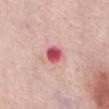* biopsy status: no biopsy performed (imaged during a skin exam)
* subject: female, in their mid-60s
* anatomic site: the front of the torso
* image source: 15 mm crop, total-body photography
* illumination: white-light
* size: about 2.5 mm